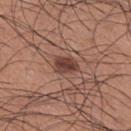Context:
A roughly 15 mm field-of-view crop from a total-body skin photograph. The subject is a male roughly 35 years of age. Automated tile analysis of the lesion measured a lesion color around L≈41 a*≈21 b*≈24 in CIELAB. And it measured radial color variation of about 1. The lesion is on the right upper arm. This is a white-light tile.